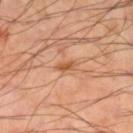Q: Was a biopsy performed?
A: no biopsy performed (imaged during a skin exam)
Q: What lighting was used for the tile?
A: cross-polarized
Q: Lesion location?
A: the leg
Q: What is the imaging modality?
A: ~15 mm tile from a whole-body skin photo
Q: How large is the lesion?
A: about 2.5 mm
Q: Automated lesion metrics?
A: an outline eccentricity of about 0.85 (0 = round, 1 = elongated) and a shape-asymmetry score of about 0.3 (0 = symmetric); a lesion color around L≈56 a*≈25 b*≈38 in CIELAB, about 10 CIELAB-L* units darker than the surrounding skin, and a lesion-to-skin contrast of about 7 (normalized; higher = more distinct); a border-irregularity index near 3/10, a within-lesion color-variation index near 0/10, and a peripheral color-asymmetry measure near 0; a classifier nevus-likeness of about 0/100 and a detector confidence of about 100 out of 100 that the crop contains a lesion
Q: What are the patient's age and sex?
A: male, in their 50s